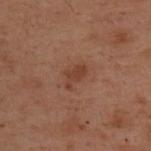Captured during whole-body skin photography for melanoma surveillance; the lesion was not biopsied. Measured at roughly 3 mm in maximum diameter. A female patient, aged around 55. The lesion is on the upper back. The lesion-visualizer software estimated a footprint of about 3.5 mm², an eccentricity of roughly 0.85, and a shape-asymmetry score of about 0.45 (0 = symmetric). A roughly 15 mm field-of-view crop from a total-body skin photograph. The tile uses cross-polarized illumination.